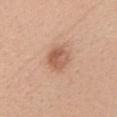The lesion was photographed on a routine skin check and not biopsied; there is no pathology result. A female subject, aged around 45. Cropped from a total-body skin-imaging series; the visible field is about 15 mm. The lesion's longest dimension is about 3 mm. The tile uses white-light illumination. From the head or neck.